A male subject, roughly 60 years of age · a 15 mm close-up tile from a total-body photography series done for melanoma screening · the lesion is on the back:
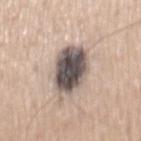Q: What did pathology find?
A: a dysplastic (Clark) nevus